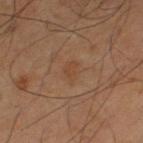Part of a total-body skin-imaging series; this lesion was reviewed on a skin check and was not flagged for biopsy.
The patient is a male about 60 years old.
From the right thigh.
Approximately 2.5 mm at its widest.
This is a cross-polarized tile.
A lesion tile, about 15 mm wide, cut from a 3D total-body photograph.